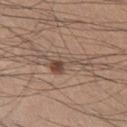notes: no biopsy performed (imaged during a skin exam) | lighting: white-light illumination | subject: male, aged approximately 35 | image: 15 mm crop, total-body photography | anatomic site: the left thigh | lesion diameter: ≈5.5 mm | automated lesion analysis: an area of roughly 7.5 mm², an outline eccentricity of about 0.95 (0 = round, 1 = elongated), and two-axis asymmetry of about 0.65; border irregularity of about 8 on a 0–10 scale and internal color variation of about 6 on a 0–10 scale.